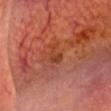Q: Was a biopsy performed?
A: no biopsy performed (imaged during a skin exam)